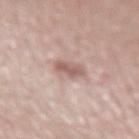| key | value |
|---|---|
| workup | no biopsy performed (imaged during a skin exam) |
| image-analysis metrics | a mean CIELAB color near L≈58 a*≈19 b*≈22 and a lesion–skin lightness drop of about 11; border irregularity of about 2 on a 0–10 scale, a color-variation rating of about 2/10, and radial color variation of about 1 |
| site | the left forearm |
| size | about 2.5 mm |
| image | ~15 mm tile from a whole-body skin photo |
| illumination | white-light |
| patient | female, aged approximately 50 |